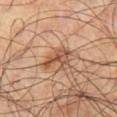Findings:
- biopsy status: total-body-photography surveillance lesion; no biopsy
- anatomic site: the leg
- size: about 4 mm
- imaging modality: ~15 mm tile from a whole-body skin photo
- tile lighting: cross-polarized
- automated lesion analysis: a lesion area of about 5.5 mm², a shape eccentricity near 0.9, and a symmetry-axis asymmetry near 0.45; an average lesion color of about L≈50 a*≈21 b*≈31 (CIELAB), a lesion–skin lightness drop of about 11, and a lesion-to-skin contrast of about 8 (normalized; higher = more distinct); a border-irregularity index near 5.5/10 and a peripheral color-asymmetry measure near 1
- patient: male, aged approximately 60